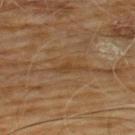Recorded during total-body skin imaging; not selected for excision or biopsy.
On the chest.
A male patient, roughly 60 years of age.
A close-up tile cropped from a whole-body skin photograph, about 15 mm across.